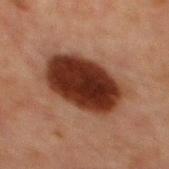* biopsy status: no biopsy performed (imaged during a skin exam)
* body site: the chest
* patient: male, approximately 65 years of age
* imaging modality: 15 mm crop, total-body photography